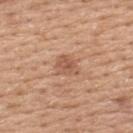biopsy_status: not biopsied; imaged during a skin examination
patient:
  sex: female
  age_approx: 55
image:
  source: total-body photography crop
  field_of_view_mm: 15
site: upper back